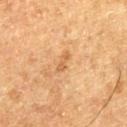The lesion was tiled from a total-body skin photograph and was not biopsied.
A male subject approximately 75 years of age.
The tile uses cross-polarized illumination.
From the leg.
A roughly 15 mm field-of-view crop from a total-body skin photograph.
The lesion's longest dimension is about 2.5 mm.